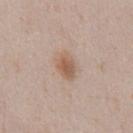<lesion>
<biopsy_status>not biopsied; imaged during a skin examination</biopsy_status>
<automated_metrics>
  <area_mm2_approx>6.0</area_mm2_approx>
  <eccentricity>0.7</eccentricity>
  <nevus_likeness_0_100>95</nevus_likeness_0_100>
  <lesion_detection_confidence_0_100>100</lesion_detection_confidence_0_100>
</automated_metrics>
<site>chest</site>
<image>
  <source>total-body photography crop</source>
  <field_of_view_mm>15</field_of_view_mm>
</image>
<patient>
  <sex>male</sex>
  <age_approx>40</age_approx>
</patient>
<lesion_size>
  <long_diameter_mm_approx>3.5</long_diameter_mm_approx>
</lesion_size>
</lesion>